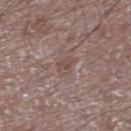Background:
The subject is a male aged approximately 50. A close-up tile cropped from a whole-body skin photograph, about 15 mm across. Located on the right lower leg.